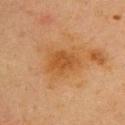{"biopsy_status": "not biopsied; imaged during a skin examination", "image": {"source": "total-body photography crop", "field_of_view_mm": 15}, "patient": {"sex": "female", "age_approx": 40}, "site": "upper back"}